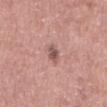Case summary:
– biopsy status — total-body-photography surveillance lesion; no biopsy
– TBP lesion metrics — an area of roughly 3 mm² and a shape eccentricity near 0.8; a lesion color around L≈53 a*≈21 b*≈22 in CIELAB, a lesion–skin lightness drop of about 11, and a normalized border contrast of about 8; a border-irregularity rating of about 2.5/10 and a color-variation rating of about 4/10
– patient — male, aged approximately 60
– acquisition — ~15 mm tile from a whole-body skin photo
– size — about 2.5 mm
– location — the mid back